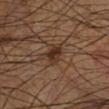Impression:
Part of a total-body skin-imaging series; this lesion was reviewed on a skin check and was not flagged for biopsy.
Background:
The tile uses cross-polarized illumination. A 15 mm crop from a total-body photograph taken for skin-cancer surveillance. The lesion is located on the left lower leg. The patient is a male aged 58 to 62. Approximately 2.5 mm at its widest.